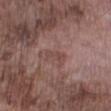This lesion was catalogued during total-body skin photography and was not selected for biopsy. From the right lower leg. The patient is a male aged 73–77. The lesion's longest dimension is about 3 mm. A close-up tile cropped from a whole-body skin photograph, about 15 mm across. An algorithmic analysis of the crop reported border irregularity of about 4.5 on a 0–10 scale, internal color variation of about 0 on a 0–10 scale, and radial color variation of about 0. The analysis additionally found a classifier nevus-likeness of about 0/100. The tile uses white-light illumination.